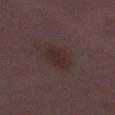  biopsy_status: not biopsied; imaged during a skin examination
  image:
    source: total-body photography crop
    field_of_view_mm: 15
  lesion_size:
    long_diameter_mm_approx: 4.0
  patient:
    sex: female
    age_approx: 30
  site: right thigh